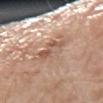Image and clinical context:
The recorded lesion diameter is about 4.5 mm. The total-body-photography lesion software estimated an average lesion color of about L≈58 a*≈18 b*≈29 (CIELAB) and a normalized lesion–skin contrast near 5.5. The software also gave an automated nevus-likeness rating near 0 out of 100 and a detector confidence of about 100 out of 100 that the crop contains a lesion. A region of skin cropped from a whole-body photographic capture, roughly 15 mm wide. From the left arm. The tile uses white-light illumination. A female patient roughly 65 years of age.